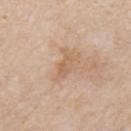Imaged during a routine full-body skin examination; the lesion was not biopsied and no histopathology is available.
Located on the left upper arm.
Captured under white-light illumination.
A male subject, roughly 85 years of age or older.
A lesion tile, about 15 mm wide, cut from a 3D total-body photograph.
Approximately 3 mm at its widest.
Automated tile analysis of the lesion measured a lesion area of about 3.5 mm², a shape eccentricity near 0.85, and a shape-asymmetry score of about 0.25 (0 = symmetric). The software also gave a lesion color around L≈61 a*≈19 b*≈32 in CIELAB and a lesion-to-skin contrast of about 5.5 (normalized; higher = more distinct). It also reported lesion-presence confidence of about 100/100.